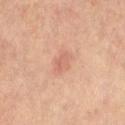Findings:
– biopsy status: imaged on a skin check; not biopsied
– subject: female, in their mid-60s
– acquisition: ~15 mm crop, total-body skin-cancer survey
– anatomic site: the abdomen
– image-analysis metrics: a lesion–skin lightness drop of about 7 and a normalized border contrast of about 4.5; an automated nevus-likeness rating near 15 out of 100 and a detector confidence of about 100 out of 100 that the crop contains a lesion